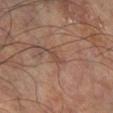Part of a total-body skin-imaging series; this lesion was reviewed on a skin check and was not flagged for biopsy.
A male subject, aged around 60.
From the right lower leg.
The total-body-photography lesion software estimated a lesion area of about 2.5 mm². The analysis additionally found an average lesion color of about L≈45 a*≈19 b*≈25 (CIELAB), roughly 7 lightness units darker than nearby skin, and a normalized border contrast of about 5.5.
Cropped from a total-body skin-imaging series; the visible field is about 15 mm.